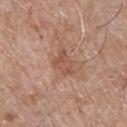  biopsy_status: not biopsied; imaged during a skin examination
  patient:
    sex: male
    age_approx: 65
  site: chest
  image:
    source: total-body photography crop
    field_of_view_mm: 15
  lighting: white-light